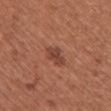The lesion was photographed on a routine skin check and not biopsied; there is no pathology result. A female patient aged around 50. Located on the chest. A 15 mm close-up tile from a total-body photography series done for melanoma screening. Imaged with white-light lighting. The recorded lesion diameter is about 3 mm.